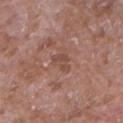{
  "biopsy_status": "not biopsied; imaged during a skin examination",
  "lighting": "white-light",
  "image": {
    "source": "total-body photography crop",
    "field_of_view_mm": 15
  },
  "patient": {
    "sex": "female",
    "age_approx": 70
  },
  "lesion_size": {
    "long_diameter_mm_approx": 2.5
  },
  "site": "right upper arm",
  "automated_metrics": {
    "cielab_L": 47,
    "cielab_a": 22,
    "cielab_b": 26,
    "vs_skin_darker_L": 7.0,
    "vs_skin_contrast_norm": 5.5
  }
}